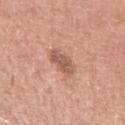workup = total-body-photography surveillance lesion; no biopsy
lesion diameter = about 3.5 mm
site = the left upper arm
image = ~15 mm crop, total-body skin-cancer survey
subject = male, aged approximately 60